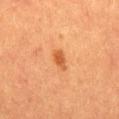Q: What are the patient's age and sex?
A: male, aged 38 to 42
Q: How large is the lesion?
A: about 2.5 mm
Q: Automated lesion metrics?
A: a footprint of about 3.5 mm² and a shape-asymmetry score of about 0.35 (0 = symmetric); a mean CIELAB color near L≈47 a*≈26 b*≈38, roughly 9 lightness units darker than nearby skin, and a normalized lesion–skin contrast near 7.5
Q: What is the imaging modality?
A: 15 mm crop, total-body photography
Q: Where on the body is the lesion?
A: the mid back